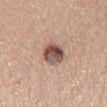workup: total-body-photography surveillance lesion; no biopsy | patient: female, aged approximately 25 | image source: ~15 mm tile from a whole-body skin photo | tile lighting: white-light illumination | lesion diameter: ≈3.5 mm | anatomic site: the head or neck | image-analysis metrics: an area of roughly 7.5 mm² and an eccentricity of roughly 0.7; a mean CIELAB color near L≈51 a*≈19 b*≈23, a lesion–skin lightness drop of about 16, and a normalized border contrast of about 11; a border-irregularity index near 1.5/10, a color-variation rating of about 8.5/10, and peripheral color asymmetry of about 3; an automated nevus-likeness rating near 90 out of 100 and a detector confidence of about 100 out of 100 that the crop contains a lesion.